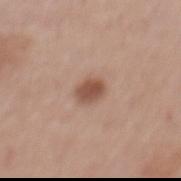notes=imaged on a skin check; not biopsied
size=about 2.5 mm
imaging modality=15 mm crop, total-body photography
patient=female, aged around 60
automated metrics=a lesion area of about 5 mm², an eccentricity of roughly 0.6, and two-axis asymmetry of about 0.15; a lesion color around L≈52 a*≈20 b*≈28 in CIELAB and a normalized border contrast of about 8.5
tile lighting=white-light
body site=the mid back